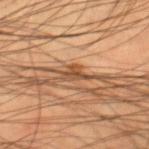| key | value |
|---|---|
| workup | catalogued during a skin exam; not biopsied |
| automated metrics | a footprint of about 3 mm², an eccentricity of roughly 0.75, and two-axis asymmetry of about 0.55; a nevus-likeness score of about 0/100 |
| patient | male, aged 43 to 47 |
| body site | the right thigh |
| imaging modality | ~15 mm crop, total-body skin-cancer survey |
| lesion diameter | about 3 mm |